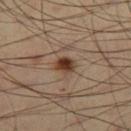workup: imaged on a skin check; not biopsied